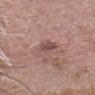workup: imaged on a skin check; not biopsied
lighting: white-light
image source: ~15 mm crop, total-body skin-cancer survey
subject: male, aged around 75
lesion diameter: ≈3 mm
automated metrics: a shape eccentricity near 0.75 and two-axis asymmetry of about 0.25; an average lesion color of about L≈49 a*≈18 b*≈20 (CIELAB), roughly 9 lightness units darker than nearby skin, and a normalized lesion–skin contrast near 7; a border-irregularity rating of about 2.5/10 and a color-variation rating of about 2/10; a lesion-detection confidence of about 100/100
location: the head or neck